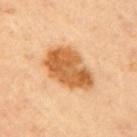Case summary:
* workup — total-body-photography surveillance lesion; no biopsy